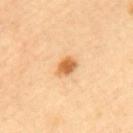The lesion was photographed on a routine skin check and not biopsied; there is no pathology result. A lesion tile, about 15 mm wide, cut from a 3D total-body photograph. The subject is a female aged approximately 60. Approximately 3 mm at its widest. From the upper back.